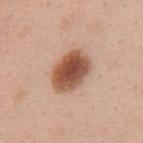biopsy status = total-body-photography surveillance lesion; no biopsy
body site = the back
subject = female, aged 48–52
size = about 5.5 mm
image = total-body-photography crop, ~15 mm field of view